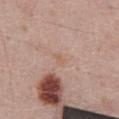No biopsy was performed on this lesion — it was imaged during a full skin examination and was not determined to be concerning. Located on the abdomen. A 15 mm close-up extracted from a 3D total-body photography capture. A male patient aged 68 to 72.